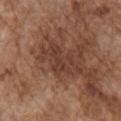This lesion was catalogued during total-body skin photography and was not selected for biopsy.
The subject is a male in their mid- to late 70s.
Imaged with white-light lighting.
The lesion is located on the right upper arm.
Measured at roughly 5.5 mm in maximum diameter.
A roughly 15 mm field-of-view crop from a total-body skin photograph.
Automated tile analysis of the lesion measured a footprint of about 10 mm², an eccentricity of roughly 0.85, and two-axis asymmetry of about 0.55.